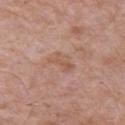Imaged during a routine full-body skin examination; the lesion was not biopsied and no histopathology is available. A lesion tile, about 15 mm wide, cut from a 3D total-body photograph. The lesion is on the left upper arm. The recorded lesion diameter is about 3 mm. The patient is a male about 65 years old.